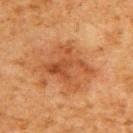follow-up: no biopsy performed (imaged during a skin exam) | tile lighting: cross-polarized | image source: 15 mm crop, total-body photography | patient: male, aged 58–62 | site: the upper back | size: about 6 mm.